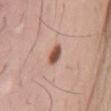Q: Was a biopsy performed?
A: catalogued during a skin exam; not biopsied
Q: How large is the lesion?
A: ≈2.5 mm
Q: What lighting was used for the tile?
A: white-light
Q: What are the patient's age and sex?
A: male, in their mid-30s
Q: What kind of image is this?
A: ~15 mm tile from a whole-body skin photo
Q: Where on the body is the lesion?
A: the mid back